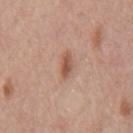notes — imaged on a skin check; not biopsied
lesion size — ~2.5 mm (longest diameter)
automated metrics — a footprint of about 3.5 mm², a shape eccentricity near 0.8, and a symmetry-axis asymmetry near 0.2; border irregularity of about 2 on a 0–10 scale, a within-lesion color-variation index near 3/10, and radial color variation of about 1
location — the back
image source — total-body-photography crop, ~15 mm field of view
subject — male, in their mid- to late 60s